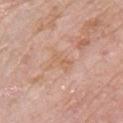No biopsy was performed on this lesion — it was imaged during a full skin examination and was not determined to be concerning.
The tile uses white-light illumination.
A lesion tile, about 15 mm wide, cut from a 3D total-body photograph.
A female subject, aged 63–67.
From the front of the torso.